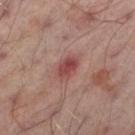Impression:
Imaged during a routine full-body skin examination; the lesion was not biopsied and no histopathology is available.
Image and clinical context:
From the left thigh. The subject is a male in their mid-60s. Measured at roughly 3 mm in maximum diameter. The tile uses cross-polarized illumination. A roughly 15 mm field-of-view crop from a total-body skin photograph.